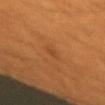<case>
  <biopsy_status>not biopsied; imaged during a skin examination</biopsy_status>
  <site>left forearm</site>
  <lighting>cross-polarized</lighting>
  <image>
    <source>total-body photography crop</source>
    <field_of_view_mm>15</field_of_view_mm>
  </image>
  <automated_metrics>
    <area_mm2_approx>1.0</area_mm2_approx>
    <eccentricity>0.8</eccentricity>
    <shape_asymmetry>0.5</shape_asymmetry>
    <vs_skin_darker_L>5.0</vs_skin_darker_L>
    <vs_skin_contrast_norm>4.0</vs_skin_contrast_norm>
    <nevus_likeness_0_100>0</nevus_likeness_0_100>
  </automated_metrics>
  <patient>
    <sex>female</sex>
    <age_approx>40</age_approx>
  </patient>
  <lesion_size>
    <long_diameter_mm_approx>1.5</long_diameter_mm_approx>
  </lesion_size>
</case>